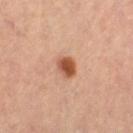Part of a total-body skin-imaging series; this lesion was reviewed on a skin check and was not flagged for biopsy. On the left thigh. Captured under cross-polarized illumination. Cropped from a total-body skin-imaging series; the visible field is about 15 mm. A female subject aged 63 to 67.